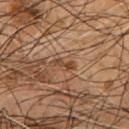Assessment: This lesion was catalogued during total-body skin photography and was not selected for biopsy. Clinical summary: A 15 mm close-up extracted from a 3D total-body photography capture. The lesion's longest dimension is about 3 mm. An algorithmic analysis of the crop reported an average lesion color of about L≈40 a*≈18 b*≈30 (CIELAB), roughly 9 lightness units darker than nearby skin, and a normalized lesion–skin contrast near 8. Imaged with cross-polarized lighting. On the chest. A male patient, aged 53–57.